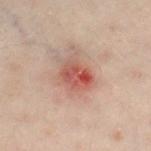follow-up = no biopsy performed (imaged during a skin exam); subject = male, aged around 50; acquisition = 15 mm crop, total-body photography; location = the left leg.